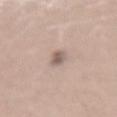workup = total-body-photography surveillance lesion; no biopsy | tile lighting = white-light | image = ~15 mm crop, total-body skin-cancer survey | location = the mid back | lesion size = ≈2.5 mm | patient = male, roughly 25 years of age.